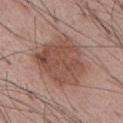Clinical impression:
The lesion was tiled from a total-body skin photograph and was not biopsied.
Background:
Located on the abdomen. Approximately 6.5 mm at its widest. The tile uses white-light illumination. A lesion tile, about 15 mm wide, cut from a 3D total-body photograph. The subject is a male aged 53 to 57.